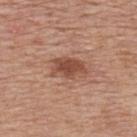workup: no biopsy performed (imaged during a skin exam)
body site: the upper back
illumination: white-light illumination
patient: male, in their 80s
acquisition: total-body-photography crop, ~15 mm field of view
lesion size: ≈4.5 mm